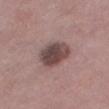Assessment:
No biopsy was performed on this lesion — it was imaged during a full skin examination and was not determined to be concerning.
Context:
The lesion is located on the leg. A close-up tile cropped from a whole-body skin photograph, about 15 mm across. Measured at roughly 4.5 mm in maximum diameter. Automated image analysis of the tile measured a footprint of about 12 mm², an outline eccentricity of about 0.7 (0 = round, 1 = elongated), and a shape-asymmetry score of about 0.15 (0 = symmetric). The analysis additionally found a lesion color around L≈45 a*≈17 b*≈18 in CIELAB, roughly 14 lightness units darker than nearby skin, and a normalized lesion–skin contrast near 10. And it measured a nevus-likeness score of about 20/100. A female patient aged approximately 45. Imaged with white-light lighting.